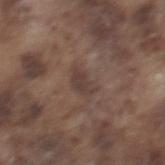Impression:
This lesion was catalogued during total-body skin photography and was not selected for biopsy.